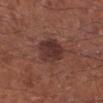Imaged during a routine full-body skin examination; the lesion was not biopsied and no histopathology is available.
On the right lower leg.
The lesion's longest dimension is about 4.5 mm.
A region of skin cropped from a whole-body photographic capture, roughly 15 mm wide.
A male patient aged around 70.
The tile uses white-light illumination.